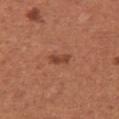Imaged during a routine full-body skin examination; the lesion was not biopsied and no histopathology is available. The lesion is on the chest. The patient is a female aged 48–52. This is a white-light tile. A close-up tile cropped from a whole-body skin photograph, about 15 mm across. Approximately 2.5 mm at its widest.